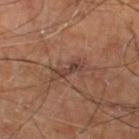image source — total-body-photography crop, ~15 mm field of view; subject — male, aged 68 to 72; site — the left lower leg.